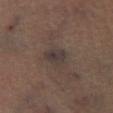Findings:
– workup — catalogued during a skin exam; not biopsied
– patient — male, aged 48 to 52
– acquisition — total-body-photography crop, ~15 mm field of view
– site — the left leg